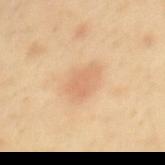{"biopsy_status": "not biopsied; imaged during a skin examination", "site": "mid back", "patient": {"sex": "male", "age_approx": 45}, "lighting": "cross-polarized", "lesion_size": {"long_diameter_mm_approx": 4.0}, "image": {"source": "total-body photography crop", "field_of_view_mm": 15}}